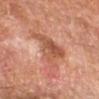Assessment:
The lesion was photographed on a routine skin check and not biopsied; there is no pathology result.
Context:
Automated image analysis of the tile measured a border-irregularity rating of about 6/10 and peripheral color asymmetry of about 2. And it measured a classifier nevus-likeness of about 0/100 and a lesion-detection confidence of about 100/100. The lesion is located on the right forearm. The patient is a male aged 68–72. A roughly 15 mm field-of-view crop from a total-body skin photograph.